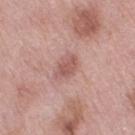* notes — total-body-photography surveillance lesion; no biopsy
* location — the right thigh
* subject — female, aged approximately 70
* diameter — ≈3 mm
* imaging modality — ~15 mm crop, total-body skin-cancer survey
* illumination — white-light
* automated metrics — an average lesion color of about L≈56 a*≈23 b*≈23 (CIELAB) and about 9 CIELAB-L* units darker than the surrounding skin; internal color variation of about 2 on a 0–10 scale